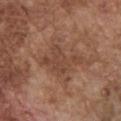Q: Is there a histopathology result?
A: imaged on a skin check; not biopsied
Q: Where on the body is the lesion?
A: the chest
Q: How large is the lesion?
A: ≈5 mm
Q: What is the imaging modality?
A: ~15 mm crop, total-body skin-cancer survey
Q: What are the patient's age and sex?
A: male, aged approximately 75